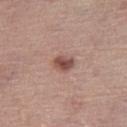Recorded during total-body skin imaging; not selected for excision or biopsy. Longest diameter approximately 2.5 mm. Captured under white-light illumination. This image is a 15 mm lesion crop taken from a total-body photograph. The lesion is located on the leg. A female subject in their mid-60s.